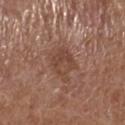Imaged during a routine full-body skin examination; the lesion was not biopsied and no histopathology is available.
An algorithmic analysis of the crop reported a lesion area of about 11 mm², an eccentricity of roughly 0.7, and a shape-asymmetry score of about 0.25 (0 = symmetric). It also reported a border-irregularity rating of about 3/10, a color-variation rating of about 3.5/10, and radial color variation of about 1.5. And it measured a classifier nevus-likeness of about 5/100 and a detector confidence of about 100 out of 100 that the crop contains a lesion.
A close-up tile cropped from a whole-body skin photograph, about 15 mm across.
This is a white-light tile.
A male patient aged approximately 75.
About 4.5 mm across.
From the right lower leg.